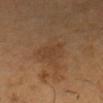No biopsy was performed on this lesion — it was imaged during a full skin examination and was not determined to be concerning. Cropped from a total-body skin-imaging series; the visible field is about 15 mm. Imaged with cross-polarized lighting. The patient is a male in their mid-40s. The lesion is on the head or neck.